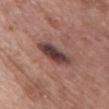The lesion was photographed on a routine skin check and not biopsied; there is no pathology result.
The lesion-visualizer software estimated an eccentricity of roughly 0.9. And it measured a border-irregularity rating of about 3/10, internal color variation of about 7 on a 0–10 scale, and a peripheral color-asymmetry measure near 2.
A region of skin cropped from a whole-body photographic capture, roughly 15 mm wide.
The patient is a female roughly 60 years of age.
From the front of the torso.
The recorded lesion diameter is about 5 mm.
The tile uses white-light illumination.